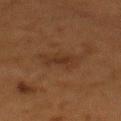Assessment:
Part of a total-body skin-imaging series; this lesion was reviewed on a skin check and was not flagged for biopsy.
Clinical summary:
Measured at roughly 3.5 mm in maximum diameter. From the mid back. This is a cross-polarized tile. A female subject, in their 50s. A roughly 15 mm field-of-view crop from a total-body skin photograph.